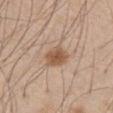follow-up: total-body-photography surveillance lesion; no biopsy | anatomic site: the right thigh | acquisition: ~15 mm crop, total-body skin-cancer survey | subject: male, aged 58–62.